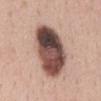Case summary:
- subject · female, about 45 years old
- acquisition · total-body-photography crop, ~15 mm field of view
- location · the front of the torso
- diameter · ≈7.5 mm
- automated lesion analysis · an area of roughly 29 mm², an eccentricity of roughly 0.8, and two-axis asymmetry of about 0.15; about 23 CIELAB-L* units darker than the surrounding skin and a normalized border contrast of about 15